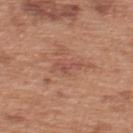Q: Lesion location?
A: the upper back
Q: What kind of image is this?
A: ~15 mm tile from a whole-body skin photo
Q: Automated lesion metrics?
A: a footprint of about 4 mm², a shape eccentricity near 0.9, and a shape-asymmetry score of about 0.4 (0 = symmetric); border irregularity of about 4.5 on a 0–10 scale and internal color variation of about 1 on a 0–10 scale; a classifier nevus-likeness of about 0/100 and a detector confidence of about 100 out of 100 that the crop contains a lesion
Q: What are the patient's age and sex?
A: male, in their mid- to late 60s